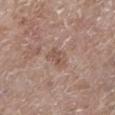Findings:
• lesion diameter · ≈3 mm
• subject · female, roughly 85 years of age or older
• image source · ~15 mm tile from a whole-body skin photo
• lighting · white-light illumination
• anatomic site · the left lower leg